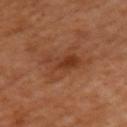Clinical impression: Imaged during a routine full-body skin examination; the lesion was not biopsied and no histopathology is available. Acquisition and patient details: A roughly 15 mm field-of-view crop from a total-body skin photograph. Captured under cross-polarized illumination. The lesion is on the left upper arm. Automated image analysis of the tile measured a lesion color around L≈41 a*≈24 b*≈33 in CIELAB and a normalized lesion–skin contrast near 6. A female subject approximately 55 years of age.